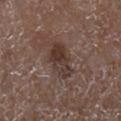Imaged during a routine full-body skin examination; the lesion was not biopsied and no histopathology is available. A female subject, aged approximately 70. The lesion's longest dimension is about 4.5 mm. Located on the right lower leg. The total-body-photography lesion software estimated a mean CIELAB color near L≈35 a*≈15 b*≈21 and a lesion–skin lightness drop of about 9. It also reported border irregularity of about 3 on a 0–10 scale, a within-lesion color-variation index near 6/10, and a peripheral color-asymmetry measure near 2. The analysis additionally found a lesion-detection confidence of about 100/100. A 15 mm close-up tile from a total-body photography series done for melanoma screening.